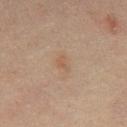  biopsy_status: not biopsied; imaged during a skin examination